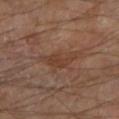Assessment:
No biopsy was performed on this lesion — it was imaged during a full skin examination and was not determined to be concerning.
Background:
A 15 mm close-up tile from a total-body photography series done for melanoma screening. From the left lower leg. The subject is a male roughly 70 years of age. Captured under cross-polarized illumination.